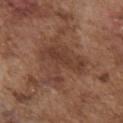Context:
The lesion is on the front of the torso. The subject is a male in their mid-70s. A 15 mm close-up extracted from a 3D total-body photography capture.